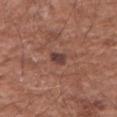* follow-up · no biopsy performed (imaged during a skin exam)
* imaging modality · ~15 mm crop, total-body skin-cancer survey
* site · the left forearm
* TBP lesion metrics · a lesion area of about 3 mm², an outline eccentricity of about 0.7 (0 = round, 1 = elongated), and two-axis asymmetry of about 0.35; a mean CIELAB color near L≈40 a*≈20 b*≈22, roughly 10 lightness units darker than nearby skin, and a normalized lesion–skin contrast near 9
* subject · male, approximately 65 years of age